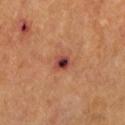The lesion was tiled from a total-body skin photograph and was not biopsied. Cropped from a whole-body photographic skin survey; the tile spans about 15 mm. A male patient about 65 years old. The lesion is located on the leg. Approximately 2.5 mm at its widest.